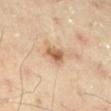<lesion>
  <patient>
    <sex>male</sex>
    <age_approx>45</age_approx>
  </patient>
  <lighting>cross-polarized</lighting>
  <automated_metrics>
    <cielab_L>51</cielab_L>
    <cielab_a>17</cielab_a>
    <cielab_b>30</cielab_b>
    <vs_skin_darker_L>11.0</vs_skin_darker_L>
    <vs_skin_contrast_norm>8.5</vs_skin_contrast_norm>
    <border_irregularity_0_10>3.0</border_irregularity_0_10>
    <color_variation_0_10>4.5</color_variation_0_10>
  </automated_metrics>
  <site>left lower leg</site>
  <image>
    <source>total-body photography crop</source>
    <field_of_view_mm>15</field_of_view_mm>
  </image>
  <lesion_size>
    <long_diameter_mm_approx>2.5</long_diameter_mm_approx>
  </lesion_size>
</lesion>